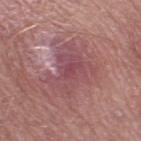| field | value |
|---|---|
| workup | total-body-photography surveillance lesion; no biopsy |